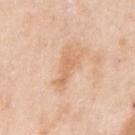Clinical impression:
This lesion was catalogued during total-body skin photography and was not selected for biopsy.
Clinical summary:
The recorded lesion diameter is about 4 mm. The lesion is on the right upper arm. A female subject, roughly 45 years of age. A 15 mm close-up extracted from a 3D total-body photography capture. The tile uses white-light illumination.